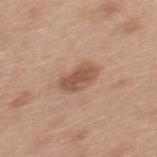biopsy status = total-body-photography surveillance lesion; no biopsy
patient = male, in their 30s
image source = ~15 mm crop, total-body skin-cancer survey
size = ~4 mm (longest diameter)
tile lighting = white-light illumination
anatomic site = the mid back